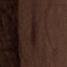<case>
<biopsy_status>not biopsied; imaged during a skin examination</biopsy_status>
<lesion_size>
  <long_diameter_mm_approx>3.5</long_diameter_mm_approx>
</lesion_size>
<patient>
  <sex>male</sex>
  <age_approx>70</age_approx>
</patient>
<image>
  <source>total-body photography crop</source>
  <field_of_view_mm>15</field_of_view_mm>
</image>
<lighting>white-light</lighting>
<automated_metrics>
  <area_mm2_approx>7.5</area_mm2_approx>
  <cielab_L>18</cielab_L>
  <cielab_a>14</cielab_a>
  <cielab_b>15</cielab_b>
  <vs_skin_contrast_norm>6.5</vs_skin_contrast_norm>
</automated_metrics>
<site>abdomen</site>
</case>